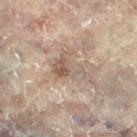Part of a total-body skin-imaging series; this lesion was reviewed on a skin check and was not flagged for biopsy. Imaged with cross-polarized lighting. Approximately 4.5 mm at its widest. A female patient aged around 80. A 15 mm crop from a total-body photograph taken for skin-cancer surveillance. On the right leg.